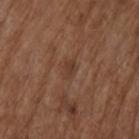A region of skin cropped from a whole-body photographic capture, roughly 15 mm wide. A male patient about 75 years old. The lesion is located on the arm. The lesion-visualizer software estimated roughly 6 lightness units darker than nearby skin and a normalized lesion–skin contrast near 5.5. The software also gave a within-lesion color-variation index near 1/10 and peripheral color asymmetry of about 0.5. The recorded lesion diameter is about 2.5 mm.